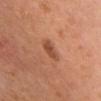The lesion-visualizer software estimated an average lesion color of about L≈51 a*≈26 b*≈34 (CIELAB) and a lesion-to-skin contrast of about 7 (normalized; higher = more distinct). And it measured a border-irregularity index near 2.5/10, a within-lesion color-variation index near 3/10, and peripheral color asymmetry of about 1. The lesion is located on the chest. The patient is a male approximately 50 years of age. Captured under white-light illumination. This image is a 15 mm lesion crop taken from a total-body photograph.